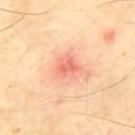notes = imaged on a skin check; not biopsied
site = the mid back
lesion size = ~3 mm (longest diameter)
image source = ~15 mm tile from a whole-body skin photo
subject = male, aged around 65